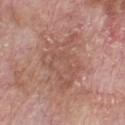Findings:
– notes: total-body-photography surveillance lesion; no biopsy
– lighting: white-light
– automated lesion analysis: an area of roughly 23 mm², an outline eccentricity of about 0.75 (0 = round, 1 = elongated), and a symmetry-axis asymmetry near 0.45; a border-irregularity rating of about 6.5/10, internal color variation of about 3 on a 0–10 scale, and peripheral color asymmetry of about 1
– patient: male, about 65 years old
– body site: the chest
– imaging modality: ~15 mm crop, total-body skin-cancer survey
– diameter: ~7.5 mm (longest diameter)